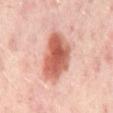Impression: Imaged during a routine full-body skin examination; the lesion was not biopsied and no histopathology is available. Acquisition and patient details: A patient in their mid- to late 50s. Imaged with cross-polarized lighting. Located on the mid back. A 15 mm close-up extracted from a 3D total-body photography capture. Approximately 6 mm at its widest.